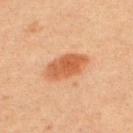Q: Was this lesion biopsied?
A: total-body-photography surveillance lesion; no biopsy
Q: Automated lesion metrics?
A: a footprint of about 11 mm² and a shape eccentricity near 0.85; a border-irregularity index near 2/10, a color-variation rating of about 3.5/10, and a peripheral color-asymmetry measure near 1; lesion-presence confidence of about 100/100
Q: What is the anatomic site?
A: the upper back
Q: How was this image acquired?
A: 15 mm crop, total-body photography
Q: How was the tile lit?
A: cross-polarized illumination
Q: How large is the lesion?
A: ≈5 mm
Q: Who is the patient?
A: male, approximately 60 years of age